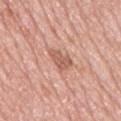Q: Was a biopsy performed?
A: catalogued during a skin exam; not biopsied